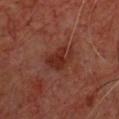Findings:
* follow-up · no biopsy performed (imaged during a skin exam)
* lesion size · about 4 mm
* lighting · cross-polarized illumination
* patient · male, aged 58–62
* site · the upper back
* imaging modality · 15 mm crop, total-body photography